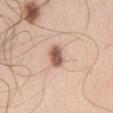• workup: no biopsy performed (imaged during a skin exam)
• lighting: white-light illumination
• anatomic site: the chest
• automated metrics: a lesion color around L≈57 a*≈21 b*≈28 in CIELAB, a lesion–skin lightness drop of about 17, and a normalized lesion–skin contrast near 10.5; a border-irregularity index near 2/10 and internal color variation of about 3.5 on a 0–10 scale; a classifier nevus-likeness of about 100/100 and a lesion-detection confidence of about 100/100
• image source: ~15 mm crop, total-body skin-cancer survey
• patient: male, about 55 years old
• lesion size: about 3 mm